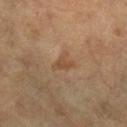Assessment:
Recorded during total-body skin imaging; not selected for excision or biopsy.
Acquisition and patient details:
From the right lower leg. Automated image analysis of the tile measured an average lesion color of about L≈46 a*≈18 b*≈31 (CIELAB), roughly 7 lightness units darker than nearby skin, and a normalized lesion–skin contrast near 5.5. And it measured radial color variation of about 0.5. Imaged with cross-polarized lighting. A roughly 15 mm field-of-view crop from a total-body skin photograph. The recorded lesion diameter is about 3 mm. A female subject, approximately 70 years of age.